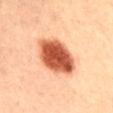Case summary:
- workup · catalogued during a skin exam; not biopsied
- lesion size · about 5 mm
- subject · male, in their 60s
- body site · the mid back
- automated metrics · an eccentricity of roughly 0.6 and a symmetry-axis asymmetry near 0.1; a border-irregularity rating of about 1.5/10, a color-variation rating of about 5.5/10, and a peripheral color-asymmetry measure near 2; an automated nevus-likeness rating near 100 out of 100
- lighting · cross-polarized illumination
- image · ~15 mm crop, total-body skin-cancer survey